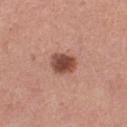Impression: This lesion was catalogued during total-body skin photography and was not selected for biopsy. Background: Automated tile analysis of the lesion measured an area of roughly 7 mm², a shape eccentricity near 0.5, and a shape-asymmetry score of about 0.2 (0 = symmetric). And it measured a within-lesion color-variation index near 3.5/10 and a peripheral color-asymmetry measure near 1. And it measured a lesion-detection confidence of about 100/100. The tile uses white-light illumination. The patient is a female aged 28–32. Located on the left thigh. A close-up tile cropped from a whole-body skin photograph, about 15 mm across.